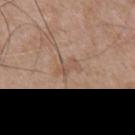Case summary:
- subject · male, aged 63–67
- location · the chest
- diameter · ≈2.5 mm
- acquisition · 15 mm crop, total-body photography